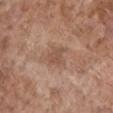Located on the chest.
The recorded lesion diameter is about 3.5 mm.
This is a white-light tile.
A region of skin cropped from a whole-body photographic capture, roughly 15 mm wide.
The lesion-visualizer software estimated a shape-asymmetry score of about 0.4 (0 = symmetric).
A male patient aged around 70.